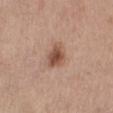This lesion was catalogued during total-body skin photography and was not selected for biopsy. The tile uses white-light illumination. Located on the right lower leg. Cropped from a whole-body photographic skin survey; the tile spans about 15 mm. A female patient, in their mid- to late 60s. The recorded lesion diameter is about 3 mm.